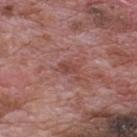* biopsy status — total-body-photography surveillance lesion; no biopsy
* TBP lesion metrics — an average lesion color of about L≈46 a*≈25 b*≈24 (CIELAB), roughly 7 lightness units darker than nearby skin, and a lesion-to-skin contrast of about 5.5 (normalized; higher = more distinct); internal color variation of about 2 on a 0–10 scale and peripheral color asymmetry of about 1
* image — ~15 mm crop, total-body skin-cancer survey
* patient — male, about 70 years old
* lesion size — ≈2.5 mm
* lighting — white-light
* location — the upper back